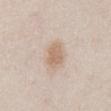Part of a total-body skin-imaging series; this lesion was reviewed on a skin check and was not flagged for biopsy.
This is a white-light tile.
From the abdomen.
A region of skin cropped from a whole-body photographic capture, roughly 15 mm wide.
A female patient approximately 40 years of age.